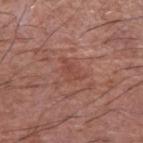Case summary:
- biopsy status — imaged on a skin check; not biopsied
- patient — male, in their mid-50s
- imaging modality — total-body-photography crop, ~15 mm field of view
- lesion size — ≈3 mm
- site — the left upper arm
- tile lighting — white-light